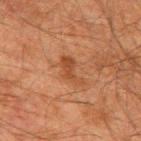The lesion was tiled from a total-body skin photograph and was not biopsied.
Measured at roughly 4 mm in maximum diameter.
Cropped from a whole-body photographic skin survey; the tile spans about 15 mm.
From the upper back.
The tile uses cross-polarized illumination.
A male patient in their 60s.